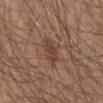A lesion tile, about 15 mm wide, cut from a 3D total-body photograph. On the abdomen. The lesion-visualizer software estimated a lesion area of about 6 mm², an outline eccentricity of about 0.75 (0 = round, 1 = elongated), and two-axis asymmetry of about 0.25. It also reported lesion-presence confidence of about 100/100. A male patient, aged 63 to 67. Approximately 3 mm at its widest.